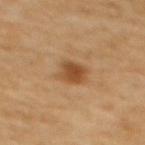This lesion was catalogued during total-body skin photography and was not selected for biopsy. A female subject, in their mid- to late 60s. Automated tile analysis of the lesion measured a footprint of about 6.5 mm² and an eccentricity of roughly 0.6. Imaged with cross-polarized lighting. A region of skin cropped from a whole-body photographic capture, roughly 15 mm wide. On the back. Measured at roughly 3.5 mm in maximum diameter.